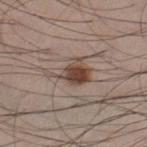Assessment:
The lesion was tiled from a total-body skin photograph and was not biopsied.
Clinical summary:
Imaged with white-light lighting. A male patient in their mid- to late 30s. Cropped from a whole-body photographic skin survey; the tile spans about 15 mm. On the left thigh. Measured at roughly 5 mm in maximum diameter.